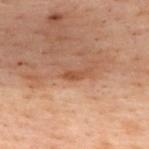Impression:
Imaged during a routine full-body skin examination; the lesion was not biopsied and no histopathology is available.
Clinical summary:
Automated tile analysis of the lesion measured an outline eccentricity of about 0.9 (0 = round, 1 = elongated) and a shape-asymmetry score of about 0.35 (0 = symmetric). The analysis additionally found border irregularity of about 3.5 on a 0–10 scale and peripheral color asymmetry of about 0. The software also gave a lesion-detection confidence of about 100/100. Longest diameter approximately 2.5 mm. A 15 mm close-up tile from a total-body photography series done for melanoma screening. Captured under cross-polarized illumination. The lesion is on the back. The patient is a female about 55 years old.